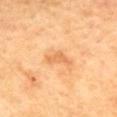Part of a total-body skin-imaging series; this lesion was reviewed on a skin check and was not flagged for biopsy. The lesion is on the mid back. A 15 mm crop from a total-body photograph taken for skin-cancer surveillance. This is a cross-polarized tile. A female patient, roughly 55 years of age. Approximately 3.5 mm at its widest.